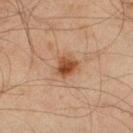{"biopsy_status": "not biopsied; imaged during a skin examination", "lesion_size": {"long_diameter_mm_approx": 3.0}, "patient": {"sex": "male", "age_approx": 45}, "image": {"source": "total-body photography crop", "field_of_view_mm": 15}, "lighting": "cross-polarized", "site": "right lower leg"}